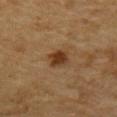Captured during whole-body skin photography for melanoma surveillance; the lesion was not biopsied.
A region of skin cropped from a whole-body photographic capture, roughly 15 mm wide.
From the mid back.
A male patient aged around 85 or older.